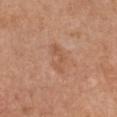notes — total-body-photography surveillance lesion; no biopsy
image-analysis metrics — a lesion area of about 5.5 mm², an eccentricity of roughly 0.85, and a shape-asymmetry score of about 0.35 (0 = symmetric); a lesion–skin lightness drop of about 6 and a lesion-to-skin contrast of about 5 (normalized; higher = more distinct); a color-variation rating of about 1.5/10; a classifier nevus-likeness of about 0/100 and a lesion-detection confidence of about 100/100
illumination — white-light illumination
imaging modality — ~15 mm crop, total-body skin-cancer survey
subject — female, roughly 55 years of age
lesion diameter — ≈3.5 mm
location — the chest